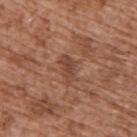biopsy status = imaged on a skin check; not biopsied | image-analysis metrics = a lesion area of about 5 mm², an eccentricity of roughly 0.85, and two-axis asymmetry of about 0.3 | size = ~3.5 mm (longest diameter) | location = the upper back | subject = male, in their mid- to late 70s | tile lighting = white-light illumination | imaging modality = total-body-photography crop, ~15 mm field of view.